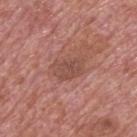Q: How was the tile lit?
A: white-light
Q: How was this image acquired?
A: ~15 mm tile from a whole-body skin photo
Q: Automated lesion metrics?
A: a lesion color around L≈49 a*≈23 b*≈26 in CIELAB, about 8 CIELAB-L* units darker than the surrounding skin, and a lesion-to-skin contrast of about 5.5 (normalized; higher = more distinct); peripheral color asymmetry of about 1; an automated nevus-likeness rating near 5 out of 100 and a detector confidence of about 100 out of 100 that the crop contains a lesion
Q: Where on the body is the lesion?
A: the upper back
Q: Patient demographics?
A: male, about 75 years old
Q: What is the lesion's diameter?
A: ≈3.5 mm